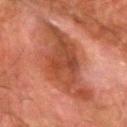Notes:
* notes — catalogued during a skin exam; not biopsied
* image source — total-body-photography crop, ~15 mm field of view
* lesion diameter — ~9.5 mm (longest diameter)
* location — the arm
* patient — male, in their 80s